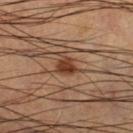follow-up — imaged on a skin check; not biopsied
location — the right lower leg
image source — 15 mm crop, total-body photography
patient — male, aged around 65